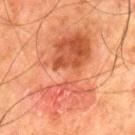Recorded during total-body skin imaging; not selected for excision or biopsy.
An algorithmic analysis of the crop reported a mean CIELAB color near L≈46 a*≈26 b*≈32. And it measured border irregularity of about 8 on a 0–10 scale, a within-lesion color-variation index near 8/10, and radial color variation of about 2.5.
The lesion's longest dimension is about 11 mm.
Located on the left thigh.
A 15 mm close-up tile from a total-body photography series done for melanoma screening.
Imaged with cross-polarized lighting.
The patient is a male roughly 80 years of age.